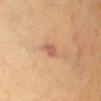Impression:
Imaged during a routine full-body skin examination; the lesion was not biopsied and no histopathology is available.
Background:
A 15 mm crop from a total-body photograph taken for skin-cancer surveillance. The patient is a female aged approximately 55. From the chest. Approximately 2.5 mm at its widest. Imaged with cross-polarized lighting.